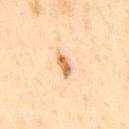This lesion was catalogued during total-body skin photography and was not selected for biopsy. A roughly 15 mm field-of-view crop from a total-body skin photograph. A male subject, about 55 years old. This is a cross-polarized tile. On the abdomen.